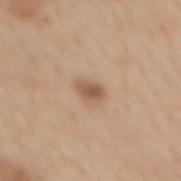| key | value |
|---|---|
| subject | female, about 65 years old |
| lesion diameter | ≈2.5 mm |
| anatomic site | the mid back |
| imaging modality | ~15 mm crop, total-body skin-cancer survey |
| lighting | white-light |